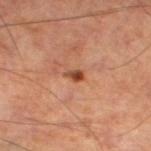No biopsy was performed on this lesion — it was imaged during a full skin examination and was not determined to be concerning. A male subject aged 53 to 57. About 2 mm across. Located on the left lower leg. A lesion tile, about 15 mm wide, cut from a 3D total-body photograph.